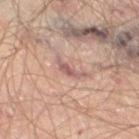Captured during whole-body skin photography for melanoma surveillance; the lesion was not biopsied.
A 15 mm close-up tile from a total-body photography series done for melanoma screening.
Imaged with cross-polarized lighting.
The recorded lesion diameter is about 3.5 mm.
A male patient, aged 63–67.
The lesion is located on the right thigh.